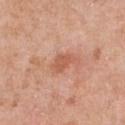workup: catalogued during a skin exam; not biopsied
patient: female, approximately 40 years of age
acquisition: ~15 mm tile from a whole-body skin photo
size: ~3 mm (longest diameter)
site: the chest
tile lighting: white-light